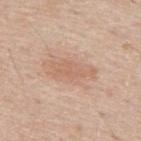Q: Who is the patient?
A: male, aged around 55
Q: How was this image acquired?
A: total-body-photography crop, ~15 mm field of view
Q: Lesion location?
A: the back
Q: Lesion size?
A: ≈5 mm
Q: What lighting was used for the tile?
A: white-light illumination